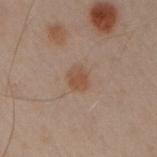Captured during whole-body skin photography for melanoma surveillance; the lesion was not biopsied. A male subject, aged 48 to 52. Captured under cross-polarized illumination. The lesion's longest dimension is about 2.5 mm. On the left arm. Cropped from a whole-body photographic skin survey; the tile spans about 15 mm.